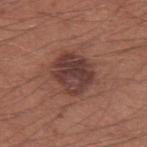The lesion was photographed on a routine skin check and not biopsied; there is no pathology result. The tile uses white-light illumination. From the left upper arm. A male subject aged approximately 35. A region of skin cropped from a whole-body photographic capture, roughly 15 mm wide.